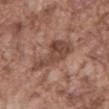The tile uses white-light illumination. On the abdomen. This image is a 15 mm lesion crop taken from a total-body photograph. A male patient, aged 73–77.